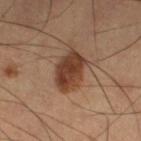<lesion>
  <biopsy_status>not biopsied; imaged during a skin examination</biopsy_status>
  <patient>
    <sex>male</sex>
    <age_approx>65</age_approx>
  </patient>
  <image>
    <source>total-body photography crop</source>
    <field_of_view_mm>15</field_of_view_mm>
  </image>
  <site>left lower leg</site>
</lesion>